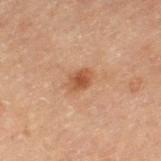Acquisition and patient details: The total-body-photography lesion software estimated an area of roughly 4.5 mm² and a symmetry-axis asymmetry near 0.25. It also reported a border-irregularity index near 2/10 and peripheral color asymmetry of about 1. The software also gave an automated nevus-likeness rating near 75 out of 100 and a lesion-detection confidence of about 100/100. A male subject aged 68 to 72. Captured under cross-polarized illumination. A 15 mm close-up tile from a total-body photography series done for melanoma screening. On the leg.